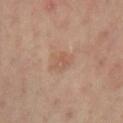Q: Was a biopsy performed?
A: catalogued during a skin exam; not biopsied
Q: What are the patient's age and sex?
A: female, aged 53–57
Q: What kind of image is this?
A: total-body-photography crop, ~15 mm field of view
Q: Lesion size?
A: about 3 mm
Q: Where on the body is the lesion?
A: the right lower leg
Q: How was the tile lit?
A: cross-polarized